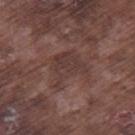Recorded during total-body skin imaging; not selected for excision or biopsy. This image is a 15 mm lesion crop taken from a total-body photograph. The patient is a male aged around 75. Measured at roughly 5 mm in maximum diameter. The lesion is located on the right thigh. An algorithmic analysis of the crop reported a shape-asymmetry score of about 0.5 (0 = symmetric). The analysis additionally found a mean CIELAB color near L≈37 a*≈18 b*≈20 and a lesion–skin lightness drop of about 6. The analysis additionally found a border-irregularity rating of about 5.5/10, a color-variation rating of about 2/10, and radial color variation of about 0.5.